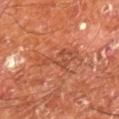Findings:
* image — ~15 mm tile from a whole-body skin photo
* automated metrics — an area of roughly 8 mm²; a mean CIELAB color near L≈47 a*≈28 b*≈34, roughly 7 lightness units darker than nearby skin, and a normalized border contrast of about 5; an automated nevus-likeness rating near 0 out of 100 and a detector confidence of about 95 out of 100 that the crop contains a lesion
* lesion size — ~5.5 mm (longest diameter)
* illumination — cross-polarized illumination
* subject — male, aged around 65
* anatomic site — the right lower leg